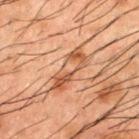Assessment:
This lesion was catalogued during total-body skin photography and was not selected for biopsy.
Acquisition and patient details:
The recorded lesion diameter is about 5.5 mm. A close-up tile cropped from a whole-body skin photograph, about 15 mm across. Captured under cross-polarized illumination. Automated image analysis of the tile measured border irregularity of about 4 on a 0–10 scale, a within-lesion color-variation index near 8/10, and radial color variation of about 2.5. A male patient about 50 years old. The lesion is on the upper back.